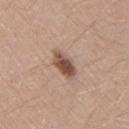biopsy_status: not biopsied; imaged during a skin examination
lighting: white-light
image:
  source: total-body photography crop
  field_of_view_mm: 15
patient:
  sex: male
  age_approx: 40
site: right upper arm
automated_metrics:
  peripheral_color_asymmetry: 1.5
  lesion_detection_confidence_0_100: 100